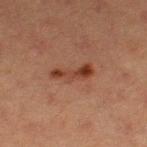About 4.5 mm across. A female subject approximately 40 years of age. The tile uses cross-polarized illumination. Located on the left thigh. A 15 mm close-up tile from a total-body photography series done for melanoma screening.